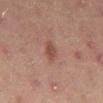The lesion was photographed on a routine skin check and not biopsied; there is no pathology result.
From the front of the torso.
A close-up tile cropped from a whole-body skin photograph, about 15 mm across.
A male subject about 45 years old.